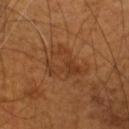Imaged during a routine full-body skin examination; the lesion was not biopsied and no histopathology is available.